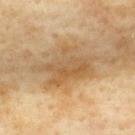notes: catalogued during a skin exam; not biopsied | location: the upper back | automated lesion analysis: an outline eccentricity of about 0.75 (0 = round, 1 = elongated); an average lesion color of about L≈52 a*≈15 b*≈36 (CIELAB), roughly 9 lightness units darker than nearby skin, and a normalized border contrast of about 7 | image source: ~15 mm crop, total-body skin-cancer survey | subject: female, approximately 60 years of age | lesion diameter: about 7 mm.